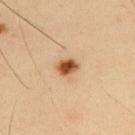Notes:
- workup — no biopsy performed (imaged during a skin exam)
- TBP lesion metrics — a footprint of about 4.5 mm² and a symmetry-axis asymmetry near 0.25; a border-irregularity rating of about 2/10, a within-lesion color-variation index near 5/10, and radial color variation of about 1.5; a classifier nevus-likeness of about 100/100 and lesion-presence confidence of about 100/100
- image source — ~15 mm crop, total-body skin-cancer survey
- anatomic site — the back
- lighting — cross-polarized illumination
- lesion diameter — ~2.5 mm (longest diameter)
- patient — male, aged 33–37